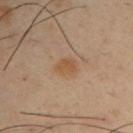Part of a total-body skin-imaging series; this lesion was reviewed on a skin check and was not flagged for biopsy. On the right upper arm. The total-body-photography lesion software estimated radial color variation of about 0.5. A male patient aged 38–42. The lesion's longest dimension is about 2.5 mm. A close-up tile cropped from a whole-body skin photograph, about 15 mm across.